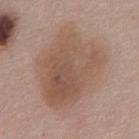Q: Patient demographics?
A: male, aged 53–57
Q: Lesion size?
A: about 9 mm
Q: Automated lesion metrics?
A: a mean CIELAB color near L≈54 a*≈17 b*≈27, roughly 9 lightness units darker than nearby skin, and a normalized border contrast of about 6.5; a border-irregularity rating of about 2.5/10, a within-lesion color-variation index near 5/10, and a peripheral color-asymmetry measure near 2; a nevus-likeness score of about 0/100 and a detector confidence of about 100 out of 100 that the crop contains a lesion
Q: Lesion location?
A: the abdomen
Q: What is the imaging modality?
A: 15 mm crop, total-body photography
Q: How was the tile lit?
A: white-light illumination